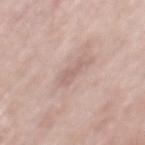Part of a total-body skin-imaging series; this lesion was reviewed on a skin check and was not flagged for biopsy.
Approximately 3 mm at its widest.
On the mid back.
A male subject roughly 55 years of age.
The lesion-visualizer software estimated a classifier nevus-likeness of about 0/100.
A 15 mm close-up tile from a total-body photography series done for melanoma screening.
The tile uses white-light illumination.